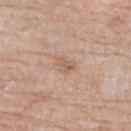The lesion was photographed on a routine skin check and not biopsied; there is no pathology result. The subject is a female aged 68–72. Measured at roughly 2.5 mm in maximum diameter. Captured under white-light illumination. The lesion-visualizer software estimated a lesion area of about 3.5 mm² and a shape-asymmetry score of about 0.4 (0 = symmetric). It also reported a mean CIELAB color near L≈59 a*≈18 b*≈30 and about 8 CIELAB-L* units darker than the surrounding skin. The software also gave a color-variation rating of about 2.5/10. Located on the leg. A close-up tile cropped from a whole-body skin photograph, about 15 mm across.